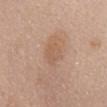  biopsy_status: not biopsied; imaged during a skin examination
  site: abdomen
  image:
    source: total-body photography crop
    field_of_view_mm: 15
  patient:
    sex: female
    age_approx: 60